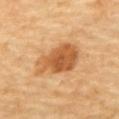notes — no biopsy performed (imaged during a skin exam) | subject — about 70 years old | image — ~15 mm crop, total-body skin-cancer survey | location — the mid back.